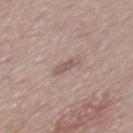Part of a total-body skin-imaging series; this lesion was reviewed on a skin check and was not flagged for biopsy. The tile uses white-light illumination. A male subject, in their mid- to late 50s. Cropped from a total-body skin-imaging series; the visible field is about 15 mm. Measured at roughly 2.5 mm in maximum diameter. The lesion is on the mid back.